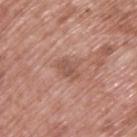Assessment: The lesion was tiled from a total-body skin photograph and was not biopsied. Acquisition and patient details: A male subject approximately 70 years of age. The lesion is located on the back. Cropped from a whole-body photographic skin survey; the tile spans about 15 mm.